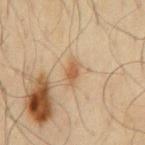Case summary:
• biopsy status: catalogued during a skin exam; not biopsied
• size: ≈3.5 mm
• subject: male, roughly 65 years of age
• tile lighting: cross-polarized illumination
• location: the mid back
• image source: ~15 mm tile from a whole-body skin photo